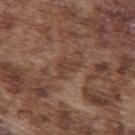{
  "biopsy_status": "not biopsied; imaged during a skin examination",
  "lesion_size": {
    "long_diameter_mm_approx": 3.0
  },
  "image": {
    "source": "total-body photography crop",
    "field_of_view_mm": 15
  },
  "patient": {
    "sex": "male",
    "age_approx": 75
  },
  "lighting": "white-light",
  "site": "upper back"
}